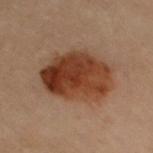- follow-up — imaged on a skin check; not biopsied